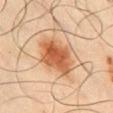{"biopsy_status": "not biopsied; imaged during a skin examination", "lighting": "cross-polarized", "automated_metrics": {"cielab_L": 55, "cielab_a": 25, "cielab_b": 38, "vs_skin_darker_L": 13.0, "vs_skin_contrast_norm": 9.5, "lesion_detection_confidence_0_100": 100}, "image": {"source": "total-body photography crop", "field_of_view_mm": 15}, "site": "chest", "patient": {"sex": "male", "age_approx": 65}, "lesion_size": {"long_diameter_mm_approx": 5.0}}